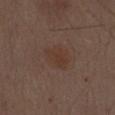Case summary:
– notes: no biopsy performed (imaged during a skin exam)
– illumination: white-light
– subject: male, roughly 70 years of age
– anatomic site: the abdomen
– image-analysis metrics: an area of roughly 9 mm², an eccentricity of roughly 0.55, and a symmetry-axis asymmetry near 0.25; a border-irregularity rating of about 2.5/10, a color-variation rating of about 2/10, and radial color variation of about 0.5; an automated nevus-likeness rating near 40 out of 100 and a detector confidence of about 100 out of 100 that the crop contains a lesion
– image: ~15 mm crop, total-body skin-cancer survey
– size: ~4 mm (longest diameter)